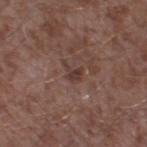notes — total-body-photography surveillance lesion; no biopsy | lesion size — ≈2.5 mm | site — the right thigh | image — total-body-photography crop, ~15 mm field of view | patient — male, aged 43–47.